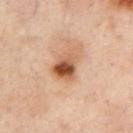Imaged during a routine full-body skin examination; the lesion was not biopsied and no histopathology is available.
This is a cross-polarized tile.
The patient is a female aged 53–57.
Located on the chest.
Automated image analysis of the tile measured a lesion area of about 8.5 mm², a shape eccentricity near 0.65, and two-axis asymmetry of about 0.3. It also reported roughly 13 lightness units darker than nearby skin and a lesion-to-skin contrast of about 9.5 (normalized; higher = more distinct). The analysis additionally found a classifier nevus-likeness of about 90/100 and a lesion-detection confidence of about 100/100.
The lesion's longest dimension is about 4 mm.
A 15 mm close-up extracted from a 3D total-body photography capture.